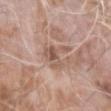The lesion was photographed on a routine skin check and not biopsied; there is no pathology result. On the left forearm. A male subject, in their mid- to late 70s. The recorded lesion diameter is about 5 mm. A roughly 15 mm field-of-view crop from a total-body skin photograph.